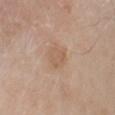Q: Was a biopsy performed?
A: catalogued during a skin exam; not biopsied
Q: How was this image acquired?
A: ~15 mm tile from a whole-body skin photo
Q: What did automated image analysis measure?
A: a footprint of about 4.5 mm², a shape eccentricity near 0.75, and two-axis asymmetry of about 0.35; border irregularity of about 3 on a 0–10 scale and a color-variation rating of about 1.5/10; a nevus-likeness score of about 0/100 and a detector confidence of about 100 out of 100 that the crop contains a lesion
Q: What lighting was used for the tile?
A: white-light illumination
Q: What is the anatomic site?
A: the chest
Q: What are the patient's age and sex?
A: male, in their mid- to late 60s